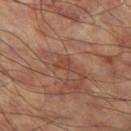| feature | finding |
|---|---|
| notes | total-body-photography surveillance lesion; no biopsy |
| patient | male, about 60 years old |
| location | the left thigh |
| acquisition | ~15 mm crop, total-body skin-cancer survey |